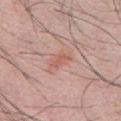<case>
<biopsy_status>not biopsied; imaged during a skin examination</biopsy_status>
<patient>
  <sex>male</sex>
  <age_approx>30</age_approx>
</patient>
<site>front of the torso</site>
<lesion_size>
  <long_diameter_mm_approx>3.0</long_diameter_mm_approx>
</lesion_size>
<automated_metrics>
  <area_mm2_approx>4.0</area_mm2_approx>
  <shape_asymmetry>0.25</shape_asymmetry>
  <vs_skin_darker_L>7.0</vs_skin_darker_L>
  <vs_skin_contrast_norm>5.5</vs_skin_contrast_norm>
  <peripheral_color_asymmetry>0.5</peripheral_color_asymmetry>
  <nevus_likeness_0_100>5</nevus_likeness_0_100>
  <lesion_detection_confidence_0_100>100</lesion_detection_confidence_0_100>
</automated_metrics>
<image>
  <source>total-body photography crop</source>
  <field_of_view_mm>15</field_of_view_mm>
</image>
</case>